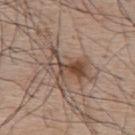Impression: This lesion was catalogued during total-body skin photography and was not selected for biopsy. Acquisition and patient details: Approximately 9 mm at its widest. The lesion is on the upper back. Automated image analysis of the tile measured a footprint of about 23 mm² and a symmetry-axis asymmetry near 0.55. And it measured a lesion color around L≈51 a*≈15 b*≈25 in CIELAB, roughly 9 lightness units darker than nearby skin, and a normalized border contrast of about 6.5. And it measured a border-irregularity rating of about 8.5/10 and radial color variation of about 3.5. This is a white-light tile. A region of skin cropped from a whole-body photographic capture, roughly 15 mm wide. A male subject in their mid- to late 60s.